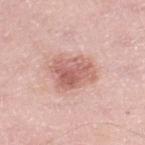Clinical impression:
No biopsy was performed on this lesion — it was imaged during a full skin examination and was not determined to be concerning.
Context:
A male patient roughly 50 years of age. On the leg. A close-up tile cropped from a whole-body skin photograph, about 15 mm across. The tile uses white-light illumination. Longest diameter approximately 5 mm. The lesion-visualizer software estimated a symmetry-axis asymmetry near 0.25. It also reported a border-irregularity rating of about 2.5/10, a color-variation rating of about 5/10, and peripheral color asymmetry of about 2. The analysis additionally found a detector confidence of about 100 out of 100 that the crop contains a lesion.